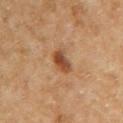{"lighting": "cross-polarized", "patient": {"sex": "female", "age_approx": 60}, "automated_metrics": {"cielab_L": 48, "cielab_a": 23, "cielab_b": 35, "vs_skin_contrast_norm": 9.0, "border_irregularity_0_10": 2.5, "color_variation_0_10": 4.0, "peripheral_color_asymmetry": 1.0, "nevus_likeness_0_100": 95, "lesion_detection_confidence_0_100": 100}, "site": "right upper arm", "image": {"source": "total-body photography crop", "field_of_view_mm": 15}}